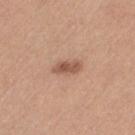{"biopsy_status": "not biopsied; imaged during a skin examination", "site": "leg", "lighting": "white-light", "image": {"source": "total-body photography crop", "field_of_view_mm": 15}, "patient": {"sex": "female", "age_approx": 45}}